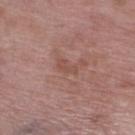{"biopsy_status": "not biopsied; imaged during a skin examination", "automated_metrics": {"eccentricity": 0.9, "shape_asymmetry": 0.5}, "site": "right lower leg", "lighting": "white-light", "patient": {"sex": "female", "age_approx": 70}, "image": {"source": "total-body photography crop", "field_of_view_mm": 15}, "lesion_size": {"long_diameter_mm_approx": 2.5}}